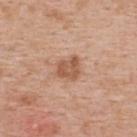Recorded during total-body skin imaging; not selected for excision or biopsy.
From the upper back.
A female subject approximately 40 years of age.
A 15 mm close-up tile from a total-body photography series done for melanoma screening.